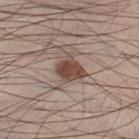No biopsy was performed on this lesion — it was imaged during a full skin examination and was not determined to be concerning. Cropped from a whole-body photographic skin survey; the tile spans about 15 mm. The subject is a male roughly 35 years of age. Longest diameter approximately 3 mm. The lesion is on the left lower leg. Captured under white-light illumination. Automated image analysis of the tile measured a lesion area of about 7 mm² and a shape eccentricity near 0.55. It also reported a nevus-likeness score of about 95/100 and lesion-presence confidence of about 100/100.